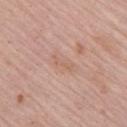The lesion was photographed on a routine skin check and not biopsied; there is no pathology result. The lesion is on the right upper arm. A lesion tile, about 15 mm wide, cut from a 3D total-body photograph. A male subject aged approximately 65. The lesion's longest dimension is about 3 mm. The tile uses white-light illumination.